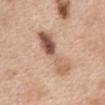The lesion was tiled from a total-body skin photograph and was not biopsied. Captured under white-light illumination. The lesion is located on the chest. A lesion tile, about 15 mm wide, cut from a 3D total-body photograph. The total-body-photography lesion software estimated a border-irregularity rating of about 5/10. A female subject, aged around 40.